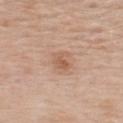{
  "biopsy_status": "not biopsied; imaged during a skin examination",
  "image": {
    "source": "total-body photography crop",
    "field_of_view_mm": 15
  },
  "automated_metrics": {
    "area_mm2_approx": 4.5,
    "eccentricity": 0.7,
    "shape_asymmetry": 0.25,
    "cielab_L": 58,
    "cielab_a": 20,
    "cielab_b": 31,
    "vs_skin_darker_L": 8.0
  },
  "patient": {
    "sex": "male",
    "age_approx": 60
  },
  "site": "upper back"
}